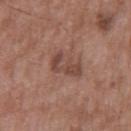* biopsy status — total-body-photography surveillance lesion; no biopsy
* patient — male, aged approximately 50
* location — the mid back
* image-analysis metrics — an area of roughly 6 mm², an outline eccentricity of about 0.85 (0 = round, 1 = elongated), and two-axis asymmetry of about 0.5
* size — ≈4 mm
* imaging modality — ~15 mm tile from a whole-body skin photo